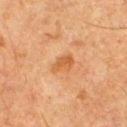{
  "biopsy_status": "not biopsied; imaged during a skin examination",
  "lighting": "cross-polarized",
  "automated_metrics": {
    "area_mm2_approx": 4.5,
    "eccentricity": 0.7,
    "shape_asymmetry": 0.3,
    "border_irregularity_0_10": 2.5,
    "color_variation_0_10": 2.0,
    "peripheral_color_asymmetry": 0.5
  },
  "site": "front of the torso",
  "image": {
    "source": "total-body photography crop",
    "field_of_view_mm": 15
  },
  "patient": {
    "sex": "male",
    "age_approx": 65
  }
}